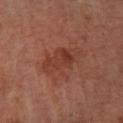Recorded during total-body skin imaging; not selected for excision or biopsy. Located on the right lower leg. The lesion-visualizer software estimated a border-irregularity index near 4.5/10 and internal color variation of about 4.5 on a 0–10 scale. A lesion tile, about 15 mm wide, cut from a 3D total-body photograph. Approximately 4.5 mm at its widest. Imaged with cross-polarized lighting. A male patient, in their mid- to late 60s.